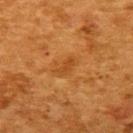About 2.5 mm across. On the upper back. The lesion-visualizer software estimated an outline eccentricity of about 0.9 (0 = round, 1 = elongated). It also reported border irregularity of about 3 on a 0–10 scale, internal color variation of about 0 on a 0–10 scale, and peripheral color asymmetry of about 0. The subject is a female aged 48 to 52. Imaged with cross-polarized lighting. A 15 mm crop from a total-body photograph taken for skin-cancer surveillance.